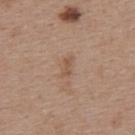Part of a total-body skin-imaging series; this lesion was reviewed on a skin check and was not flagged for biopsy.
Cropped from a total-body skin-imaging series; the visible field is about 15 mm.
The recorded lesion diameter is about 2.5 mm.
A female patient aged approximately 40.
On the upper back.